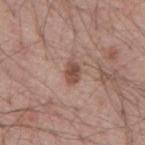Assessment:
Captured during whole-body skin photography for melanoma surveillance; the lesion was not biopsied.
Clinical summary:
The patient is a male roughly 55 years of age. Measured at roughly 2.5 mm in maximum diameter. The lesion-visualizer software estimated a lesion–skin lightness drop of about 12 and a normalized border contrast of about 8.5. From the mid back. A region of skin cropped from a whole-body photographic capture, roughly 15 mm wide.